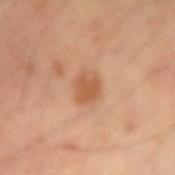| feature | finding |
|---|---|
| workup | no biopsy performed (imaged during a skin exam) |
| lighting | cross-polarized |
| subject | male, approximately 50 years of age |
| imaging modality | total-body-photography crop, ~15 mm field of view |
| body site | the right upper arm |
| lesion diameter | ~3 mm (longest diameter) |